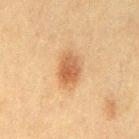diameter: ~4 mm (longest diameter)
body site: the leg
automated lesion analysis: a lesion area of about 8 mm² and a symmetry-axis asymmetry near 0.2; a lesion color around L≈54 a*≈21 b*≈36 in CIELAB, about 11 CIELAB-L* units darker than the surrounding skin, and a lesion-to-skin contrast of about 7.5 (normalized; higher = more distinct); border irregularity of about 2 on a 0–10 scale and a color-variation rating of about 3.5/10; a classifier nevus-likeness of about 100/100 and a detector confidence of about 100 out of 100 that the crop contains a lesion
imaging modality: total-body-photography crop, ~15 mm field of view
lighting: cross-polarized illumination
patient: female, in their mid-50s